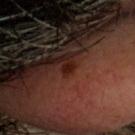Notes:
- biopsy status · no biopsy performed (imaged during a skin exam)
- patient · male, about 40 years old
- image source · ~15 mm crop, total-body skin-cancer survey
- body site · the head or neck